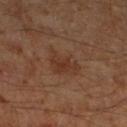Recorded during total-body skin imaging; not selected for excision or biopsy. The lesion is on the left lower leg. The patient is a male approximately 60 years of age. A 15 mm close-up tile from a total-body photography series done for melanoma screening.